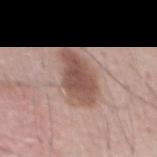Case summary:
- biopsy status · catalogued during a skin exam; not biopsied
- subject · male, aged 53–57
- TBP lesion metrics · an eccentricity of roughly 0.85 and a symmetry-axis asymmetry near 0.25; a lesion color around L≈52 a*≈19 b*≈24 in CIELAB, a lesion–skin lightness drop of about 13, and a normalized border contrast of about 9; a border-irregularity index near 2.5/10 and radial color variation of about 1; an automated nevus-likeness rating near 85 out of 100 and a detector confidence of about 100 out of 100 that the crop contains a lesion
- lighting · white-light illumination
- image source · total-body-photography crop, ~15 mm field of view